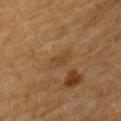The lesion was tiled from a total-body skin photograph and was not biopsied. A 15 mm crop from a total-body photograph taken for skin-cancer surveillance. From the front of the torso. The subject is a male in their mid- to late 60s. The total-body-photography lesion software estimated a normalized lesion–skin contrast near 5. The software also gave a within-lesion color-variation index near 1.5/10. And it measured a classifier nevus-likeness of about 0/100 and a lesion-detection confidence of about 95/100. The tile uses cross-polarized illumination. The lesion's longest dimension is about 3.5 mm.